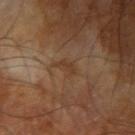| feature | finding |
|---|---|
| notes | catalogued during a skin exam; not biopsied |
| automated metrics | a normalized border contrast of about 5; a nevus-likeness score of about 0/100 and a lesion-detection confidence of about 100/100 |
| diameter | about 3 mm |
| body site | the right upper arm |
| subject | male, about 70 years old |
| imaging modality | ~15 mm tile from a whole-body skin photo |
| lighting | cross-polarized |